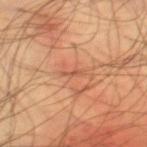Q: Was this lesion biopsied?
A: total-body-photography surveillance lesion; no biopsy
Q: Who is the patient?
A: male, in their mid- to late 40s
Q: What is the lesion's diameter?
A: about 2.5 mm
Q: What did automated image analysis measure?
A: a nevus-likeness score of about 0/100 and lesion-presence confidence of about 80/100
Q: What lighting was used for the tile?
A: cross-polarized illumination
Q: How was this image acquired?
A: total-body-photography crop, ~15 mm field of view
Q: What is the anatomic site?
A: the right lower leg